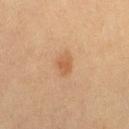workup=total-body-photography surveillance lesion; no biopsy
image=15 mm crop, total-body photography
subject=female, aged approximately 50
location=the right thigh